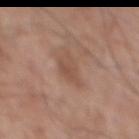notes: imaged on a skin check; not biopsied | TBP lesion metrics: a lesion area of about 6 mm², an outline eccentricity of about 0.65 (0 = round, 1 = elongated), and two-axis asymmetry of about 0.3; a lesion color around L≈51 a*≈19 b*≈28 in CIELAB, about 7 CIELAB-L* units darker than the surrounding skin, and a lesion-to-skin contrast of about 5.5 (normalized; higher = more distinct); border irregularity of about 3.5 on a 0–10 scale and radial color variation of about 1; a classifier nevus-likeness of about 0/100 | patient: male, aged 68–72 | illumination: white-light | lesion size: ~3.5 mm (longest diameter) | image source: 15 mm crop, total-body photography | body site: the abdomen.